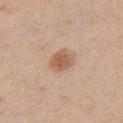Assessment:
This lesion was catalogued during total-body skin photography and was not selected for biopsy.
Image and clinical context:
From the left upper arm. A male subject aged approximately 55. This image is a 15 mm lesion crop taken from a total-body photograph.